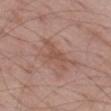Impression: Recorded during total-body skin imaging; not selected for excision or biopsy. Context: The lesion is located on the leg. Approximately 5 mm at its widest. Cropped from a whole-body photographic skin survey; the tile spans about 15 mm. The patient is a male in their mid- to late 50s.